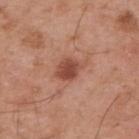Clinical impression:
This lesion was catalogued during total-body skin photography and was not selected for biopsy.
Acquisition and patient details:
The recorded lesion diameter is about 4 mm. Cropped from a whole-body photographic skin survey; the tile spans about 15 mm. On the upper back. The subject is a male in their mid- to late 50s. The lesion-visualizer software estimated a lesion area of about 7.5 mm² and a symmetry-axis asymmetry near 0.2. It also reported a nevus-likeness score of about 85/100 and lesion-presence confidence of about 100/100. Imaged with white-light lighting.